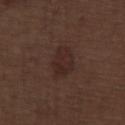From the right lower leg.
A male subject, approximately 70 years of age.
Automated image analysis of the tile measured an outline eccentricity of about 0.75 (0 = round, 1 = elongated). The analysis additionally found a border-irregularity index near 1.5/10, internal color variation of about 2.5 on a 0–10 scale, and peripheral color asymmetry of about 1.
Captured under white-light illumination.
Cropped from a total-body skin-imaging series; the visible field is about 15 mm.
The lesion's longest dimension is about 3.5 mm.